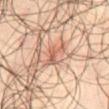This is a cross-polarized tile.
A 15 mm close-up extracted from a 3D total-body photography capture.
The lesion is on the abdomen.
Automated tile analysis of the lesion measured a lesion area of about 4 mm², an outline eccentricity of about 0.75 (0 = round, 1 = elongated), and a symmetry-axis asymmetry near 0.65. And it measured a border-irregularity rating of about 6.5/10, internal color variation of about 2.5 on a 0–10 scale, and radial color variation of about 0. And it measured an automated nevus-likeness rating near 0 out of 100.
The recorded lesion diameter is about 3 mm.
The subject is a male in their mid- to late 60s.